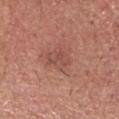{
  "biopsy_status": "not biopsied; imaged during a skin examination",
  "patient": {
    "sex": "male",
    "age_approx": 75
  },
  "site": "head or neck",
  "image": {
    "source": "total-body photography crop",
    "field_of_view_mm": 15
  },
  "lesion_size": {
    "long_diameter_mm_approx": 3.0
  },
  "lighting": "white-light",
  "automated_metrics": {
    "color_variation_0_10": 0.0,
    "peripheral_color_asymmetry": 0.0
  }
}